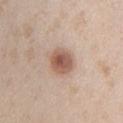Recorded during total-body skin imaging; not selected for excision or biopsy. Automated tile analysis of the lesion measured an area of roughly 8 mm², an outline eccentricity of about 0.45 (0 = round, 1 = elongated), and two-axis asymmetry of about 0.15. The software also gave a border-irregularity rating of about 1/10, a within-lesion color-variation index near 4.5/10, and peripheral color asymmetry of about 1. And it measured a classifier nevus-likeness of about 100/100 and a detector confidence of about 100 out of 100 that the crop contains a lesion. On the upper back. Imaged with white-light lighting. This image is a 15 mm lesion crop taken from a total-body photograph. A female subject, aged 23–27.